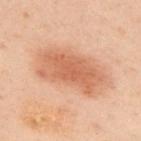The lesion was photographed on a routine skin check and not biopsied; there is no pathology result.
Cropped from a whole-body photographic skin survey; the tile spans about 15 mm.
Imaged with cross-polarized lighting.
A male subject, aged approximately 50.
Located on the upper back.